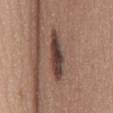| key | value |
|---|---|
| workup | imaged on a skin check; not biopsied |
| subject | female, about 45 years old |
| acquisition | 15 mm crop, total-body photography |
| body site | the mid back |
| automated lesion analysis | a shape eccentricity near 0.95 and a shape-asymmetry score of about 0.2 (0 = symmetric); an average lesion color of about L≈41 a*≈17 b*≈22 (CIELAB) and a lesion–skin lightness drop of about 14 |
| lesion diameter | ~6 mm (longest diameter) |
| illumination | white-light |